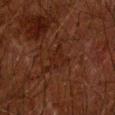Case summary:
– image source: 15 mm crop, total-body photography
– lesion size: ~3 mm (longest diameter)
– location: the arm
– illumination: cross-polarized illumination
– subject: male, in their 60s